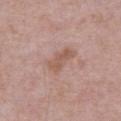Captured during whole-body skin photography for melanoma surveillance; the lesion was not biopsied. Located on the abdomen. This is a white-light tile. Cropped from a whole-body photographic skin survey; the tile spans about 15 mm. About 4 mm across. A male subject, aged approximately 65. An algorithmic analysis of the crop reported a footprint of about 6 mm² and a shape eccentricity near 0.9. The analysis additionally found a lesion color around L≈56 a*≈20 b*≈26 in CIELAB, a lesion–skin lightness drop of about 8, and a normalized border contrast of about 6.5. And it measured a border-irregularity rating of about 3.5/10, internal color variation of about 2.5 on a 0–10 scale, and a peripheral color-asymmetry measure near 1. The analysis additionally found an automated nevus-likeness rating near 0 out of 100 and a detector confidence of about 100 out of 100 that the crop contains a lesion.